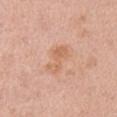image source: ~15 mm crop, total-body skin-cancer survey; tile lighting: white-light illumination; site: the right upper arm; subject: female, roughly 45 years of age; diameter: ~4 mm (longest diameter).